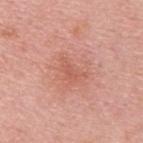workup: total-body-photography surveillance lesion; no biopsy
location: the upper back
lesion diameter: ≈3 mm
acquisition: 15 mm crop, total-body photography
patient: male, approximately 65 years of age
TBP lesion metrics: a lesion color around L≈58 a*≈28 b*≈31 in CIELAB, about 6 CIELAB-L* units darker than the surrounding skin, and a normalized border contrast of about 4.5
illumination: white-light